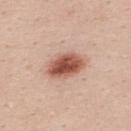Impression: This lesion was catalogued during total-body skin photography and was not selected for biopsy. Acquisition and patient details: A female patient, aged approximately 20. Cropped from a whole-body photographic skin survey; the tile spans about 15 mm. Located on the upper back. The lesion-visualizer software estimated a lesion area of about 10 mm² and an eccentricity of roughly 0.75. The analysis additionally found a classifier nevus-likeness of about 100/100 and a lesion-detection confidence of about 100/100. Measured at roughly 4.5 mm in maximum diameter.